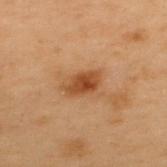Part of a total-body skin-imaging series; this lesion was reviewed on a skin check and was not flagged for biopsy. A male patient in their mid-50s. The lesion is located on the upper back. A roughly 15 mm field-of-view crop from a total-body skin photograph.